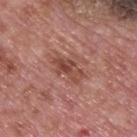| key | value |
|---|---|
| notes | catalogued during a skin exam; not biopsied |
| site | the upper back |
| diameter | ≈4 mm |
| subject | male, about 75 years old |
| acquisition | 15 mm crop, total-body photography |
| illumination | white-light |